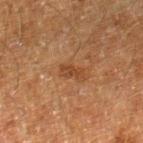Impression:
No biopsy was performed on this lesion — it was imaged during a full skin examination and was not determined to be concerning.
Background:
The lesion is on the right lower leg. A 15 mm close-up tile from a total-body photography series done for melanoma screening. A male subject, aged around 60.